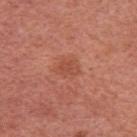Assessment: No biopsy was performed on this lesion — it was imaged during a full skin examination and was not determined to be concerning. Acquisition and patient details: Cropped from a whole-body photographic skin survey; the tile spans about 15 mm. The patient is a female approximately 50 years of age. Located on the left upper arm.